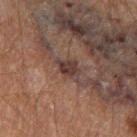Q: Was a biopsy performed?
A: imaged on a skin check; not biopsied